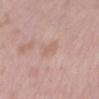Q: Is there a histopathology result?
A: catalogued during a skin exam; not biopsied
Q: What lighting was used for the tile?
A: white-light
Q: Automated lesion metrics?
A: a lesion area of about 3 mm², an eccentricity of roughly 0.85, and a shape-asymmetry score of about 0.3 (0 = symmetric); a lesion color around L≈62 a*≈20 b*≈26 in CIELAB, roughly 6 lightness units darker than nearby skin, and a normalized lesion–skin contrast near 4.5; a border-irregularity rating of about 3/10, internal color variation of about 1 on a 0–10 scale, and peripheral color asymmetry of about 0.5; an automated nevus-likeness rating near 0 out of 100 and a detector confidence of about 100 out of 100 that the crop contains a lesion
Q: How was this image acquired?
A: ~15 mm tile from a whole-body skin photo
Q: How large is the lesion?
A: about 2.5 mm
Q: What are the patient's age and sex?
A: male, aged around 55
Q: What is the anatomic site?
A: the right upper arm